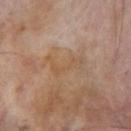Notes:
– follow-up — total-body-photography surveillance lesion; no biopsy
– patient — male, aged 68 to 72
– location — the left thigh
– image — total-body-photography crop, ~15 mm field of view
– size — ≈4.5 mm